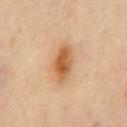– notes: total-body-photography surveillance lesion; no biopsy
– size: ~4.5 mm (longest diameter)
– acquisition: ~15 mm tile from a whole-body skin photo
– tile lighting: cross-polarized
– site: the chest